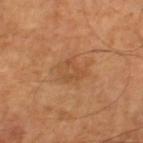follow-up: imaged on a skin check; not biopsied | subject: male, aged around 65 | lesion diameter: ≈3.5 mm | lighting: cross-polarized illumination | image-analysis metrics: an area of roughly 7.5 mm² and an outline eccentricity of about 0.6 (0 = round, 1 = elongated); a classifier nevus-likeness of about 5/100 and lesion-presence confidence of about 100/100 | image source: total-body-photography crop, ~15 mm field of view.